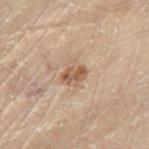Case summary:
• biopsy status · catalogued during a skin exam; not biopsied
• subject · male, in their 70s
• size · ~3 mm (longest diameter)
• image-analysis metrics · an average lesion color of about L≈41 a*≈14 b*≈25 (CIELAB), about 9 CIELAB-L* units darker than the surrounding skin, and a normalized border contrast of about 8; an automated nevus-likeness rating near 45 out of 100
• illumination · cross-polarized
• image · ~15 mm crop, total-body skin-cancer survey
• location · the left lower leg